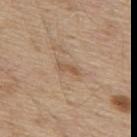Impression: Captured during whole-body skin photography for melanoma surveillance; the lesion was not biopsied. Acquisition and patient details: The total-body-photography lesion software estimated a shape eccentricity near 0.95 and a symmetry-axis asymmetry near 0.35. The analysis additionally found a normalized border contrast of about 6. And it measured a border-irregularity rating of about 4/10, a color-variation rating of about 0/10, and peripheral color asymmetry of about 0. Measured at roughly 2.5 mm in maximum diameter. The lesion is located on the mid back. The tile uses white-light illumination. A male subject aged approximately 70. A 15 mm crop from a total-body photograph taken for skin-cancer surveillance.